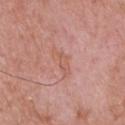No biopsy was performed on this lesion — it was imaged during a full skin examination and was not determined to be concerning. On the chest. The subject is a male aged around 50. Cropped from a total-body skin-imaging series; the visible field is about 15 mm.